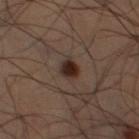The lesion was tiled from a total-body skin photograph and was not biopsied.
Longest diameter approximately 2.5 mm.
The lesion-visualizer software estimated a footprint of about 4.5 mm² and a symmetry-axis asymmetry near 0.25. It also reported border irregularity of about 2 on a 0–10 scale, a color-variation rating of about 3/10, and a peripheral color-asymmetry measure near 1. And it measured a classifier nevus-likeness of about 100/100 and a lesion-detection confidence of about 100/100.
The subject is a male aged approximately 65.
This image is a 15 mm lesion crop taken from a total-body photograph.
The lesion is located on the left thigh.
Captured under cross-polarized illumination.